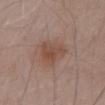biopsy_status: not biopsied; imaged during a skin examination
lesion_size:
  long_diameter_mm_approx: 4.0
image:
  source: total-body photography crop
  field_of_view_mm: 15
site: mid back
lighting: white-light
patient:
  sex: male
  age_approx: 55